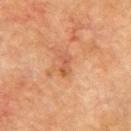Case summary:
– biopsy status — no biopsy performed (imaged during a skin exam)
– image — total-body-photography crop, ~15 mm field of view
– illumination — cross-polarized illumination
– size — about 2.5 mm
– patient — male, approximately 75 years of age
– anatomic site — the right forearm
– image-analysis metrics — an average lesion color of about L≈46 a*≈23 b*≈32 (CIELAB), roughly 7 lightness units darker than nearby skin, and a lesion-to-skin contrast of about 5.5 (normalized; higher = more distinct); border irregularity of about 3 on a 0–10 scale and peripheral color asymmetry of about 0